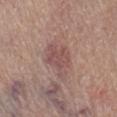{"biopsy_status": "not biopsied; imaged during a skin examination", "lighting": "white-light", "image": {"source": "total-body photography crop", "field_of_view_mm": 15}, "lesion_size": {"long_diameter_mm_approx": 5.5}, "site": "abdomen", "patient": {"sex": "male", "age_approx": 80}}